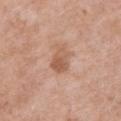Imaged during a routine full-body skin examination; the lesion was not biopsied and no histopathology is available. On the chest. A female patient, aged approximately 40. The recorded lesion diameter is about 4 mm. A close-up tile cropped from a whole-body skin photograph, about 15 mm across. This is a white-light tile.